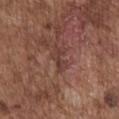* notes: no biopsy performed (imaged during a skin exam)
* site: the front of the torso
* illumination: white-light
* subject: male, aged 73–77
* image source: total-body-photography crop, ~15 mm field of view